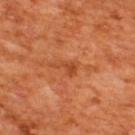Assessment:
No biopsy was performed on this lesion — it was imaged during a full skin examination and was not determined to be concerning.
Background:
A 15 mm close-up tile from a total-body photography series done for melanoma screening. Located on the upper back. A male patient, in their mid-60s.